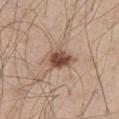Recorded during total-body skin imaging; not selected for excision or biopsy. A male subject, roughly 60 years of age. On the right thigh. The recorded lesion diameter is about 3.5 mm. Captured under white-light illumination. Automated tile analysis of the lesion measured an area of roughly 7 mm², an outline eccentricity of about 0.75 (0 = round, 1 = elongated), and a shape-asymmetry score of about 0.25 (0 = symmetric). It also reported a mean CIELAB color near L≈49 a*≈19 b*≈27, a lesion–skin lightness drop of about 16, and a lesion-to-skin contrast of about 11 (normalized; higher = more distinct). It also reported a border-irregularity rating of about 2.5/10, internal color variation of about 4.5 on a 0–10 scale, and radial color variation of about 1.5. It also reported lesion-presence confidence of about 100/100. A 15 mm close-up extracted from a 3D total-body photography capture.